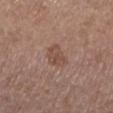This lesion was catalogued during total-body skin photography and was not selected for biopsy. This is a white-light tile. About 3 mm across. A female subject, in their mid-60s. On the left lower leg. A roughly 15 mm field-of-view crop from a total-body skin photograph.